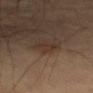Impression:
This lesion was catalogued during total-body skin photography and was not selected for biopsy.
Background:
On the left thigh. Approximately 3.5 mm at its widest. Automated image analysis of the tile measured an area of roughly 4 mm² and an eccentricity of roughly 0.85. And it measured a lesion color around L≈32 a*≈15 b*≈23 in CIELAB, a lesion–skin lightness drop of about 5, and a lesion-to-skin contrast of about 5.5 (normalized; higher = more distinct). A close-up tile cropped from a whole-body skin photograph, about 15 mm across. The subject is aged 63 to 67.